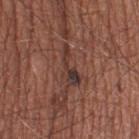Case summary:
• workup: imaged on a skin check; not biopsied
• subject: male, approximately 55 years of age
• site: the right thigh
• image source: total-body-photography crop, ~15 mm field of view